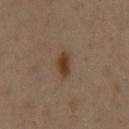Part of a total-body skin-imaging series; this lesion was reviewed on a skin check and was not flagged for biopsy.
The lesion is on the front of the torso.
Captured under cross-polarized illumination.
Automated tile analysis of the lesion measured a lesion color around L≈33 a*≈16 b*≈27 in CIELAB and about 10 CIELAB-L* units darker than the surrounding skin.
A close-up tile cropped from a whole-body skin photograph, about 15 mm across.
The lesion's longest dimension is about 3 mm.
A male subject approximately 60 years of age.